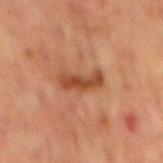Impression: Recorded during total-body skin imaging; not selected for excision or biopsy. Image and clinical context: This is a cross-polarized tile. Approximately 4 mm at its widest. The lesion is located on the mid back. A male subject in their 60s. Cropped from a total-body skin-imaging series; the visible field is about 15 mm.